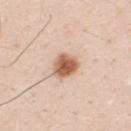Q: Is there a histopathology result?
A: total-body-photography surveillance lesion; no biopsy
Q: Automated lesion metrics?
A: an area of roughly 7 mm², a shape eccentricity near 0.6, and a shape-asymmetry score of about 0.2 (0 = symmetric); an average lesion color of about L≈61 a*≈22 b*≈33 (CIELAB) and a lesion–skin lightness drop of about 17; a classifier nevus-likeness of about 100/100 and lesion-presence confidence of about 100/100
Q: What is the imaging modality?
A: ~15 mm crop, total-body skin-cancer survey
Q: Lesion location?
A: the mid back
Q: Who is the patient?
A: male, in their mid- to late 30s
Q: How large is the lesion?
A: ~3 mm (longest diameter)
Q: How was the tile lit?
A: white-light